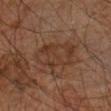Assessment: No biopsy was performed on this lesion — it was imaged during a full skin examination and was not determined to be concerning. Context: The lesion is located on the left arm. A close-up tile cropped from a whole-body skin photograph, about 15 mm across. The patient is a male about 65 years old. The lesion-visualizer software estimated border irregularity of about 2 on a 0–10 scale, internal color variation of about 4 on a 0–10 scale, and radial color variation of about 1.5. The software also gave a nevus-likeness score of about 0/100. Measured at roughly 5 mm in maximum diameter.